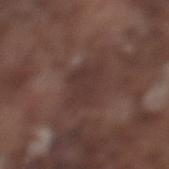Findings:
• follow-up — no biopsy performed (imaged during a skin exam)
• subject — male, aged 73 to 77
• lesion size — ≈3.5 mm
• body site — the left lower leg
• tile lighting — white-light illumination
• imaging modality — ~15 mm crop, total-body skin-cancer survey
• image-analysis metrics — two-axis asymmetry of about 0.3; border irregularity of about 3.5 on a 0–10 scale and a within-lesion color-variation index near 1.5/10